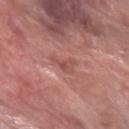Notes:
- workup — total-body-photography surveillance lesion; no biopsy
- size — ≈2.5 mm
- illumination — white-light illumination
- site — the left forearm
- patient — female, aged 58–62
- image — total-body-photography crop, ~15 mm field of view